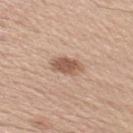Findings:
- workup · catalogued during a skin exam; not biopsied
- location · the right upper arm
- subject · male, about 30 years old
- image-analysis metrics · an area of roughly 6 mm², an outline eccentricity of about 0.85 (0 = round, 1 = elongated), and a shape-asymmetry score of about 0.25 (0 = symmetric); roughly 13 lightness units darker than nearby skin and a lesion-to-skin contrast of about 8.5 (normalized; higher = more distinct); a classifier nevus-likeness of about 95/100 and a lesion-detection confidence of about 100/100
- imaging modality · total-body-photography crop, ~15 mm field of view